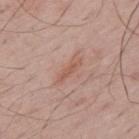  biopsy_status: not biopsied; imaged during a skin examination
  lighting: white-light
  lesion_size:
    long_diameter_mm_approx: 4.0
  site: upper back
  patient:
    sex: male
    age_approx: 65
  image:
    source: total-body photography crop
    field_of_view_mm: 15